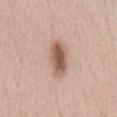The lesion is located on the front of the torso. Captured under white-light illumination. Cropped from a total-body skin-imaging series; the visible field is about 15 mm. The patient is a female in their 40s. The lesion's longest dimension is about 5 mm.